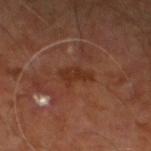{
  "biopsy_status": "not biopsied; imaged during a skin examination",
  "patient": {
    "sex": "male",
    "age_approx": 60
  },
  "lighting": "cross-polarized",
  "lesion_size": {
    "long_diameter_mm_approx": 3.5
  },
  "image": {
    "source": "total-body photography crop",
    "field_of_view_mm": 15
  },
  "site": "right leg"
}